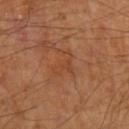Part of a total-body skin-imaging series; this lesion was reviewed on a skin check and was not flagged for biopsy.
Approximately 3.5 mm at its widest.
A 15 mm crop from a total-body photograph taken for skin-cancer surveillance.
From the arm.
This is a cross-polarized tile.